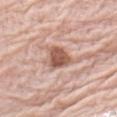follow-up: catalogued during a skin exam; not biopsied
automated metrics: border irregularity of about 3.5 on a 0–10 scale and radial color variation of about 1.5; a nevus-likeness score of about 80/100 and lesion-presence confidence of about 100/100
lesion diameter: ≈3.5 mm
image source: 15 mm crop, total-body photography
subject: female, in their mid- to late 60s
body site: the left upper arm
lighting: white-light